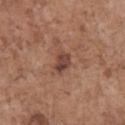Part of a total-body skin-imaging series; this lesion was reviewed on a skin check and was not flagged for biopsy.
About 3 mm across.
The patient is a male aged around 70.
Automated image analysis of the tile measured a lesion area of about 4.5 mm², an outline eccentricity of about 0.7 (0 = round, 1 = elongated), and two-axis asymmetry of about 0.3. The analysis additionally found roughly 10 lightness units darker than nearby skin and a normalized border contrast of about 8.5. And it measured internal color variation of about 4.5 on a 0–10 scale and a peripheral color-asymmetry measure near 1.5.
On the chest.
A 15 mm crop from a total-body photograph taken for skin-cancer surveillance.
Imaged with white-light lighting.